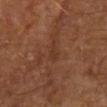<case>
  <patient>
    <sex>male</sex>
    <age_approx>70</age_approx>
  </patient>
  <image>
    <source>total-body photography crop</source>
    <field_of_view_mm>15</field_of_view_mm>
  </image>
  <site>right upper arm</site>
</case>